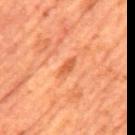This lesion was catalogued during total-body skin photography and was not selected for biopsy. Automated image analysis of the tile measured a normalized border contrast of about 7. The analysis additionally found a color-variation rating of about 0/10. It also reported an automated nevus-likeness rating near 5 out of 100 and a lesion-detection confidence of about 100/100. The lesion's longest dimension is about 2.5 mm. A male patient, roughly 65 years of age. Captured under cross-polarized illumination. A 15 mm crop from a total-body photograph taken for skin-cancer surveillance. On the mid back.